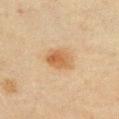follow-up = imaged on a skin check; not biopsied
TBP lesion metrics = a mean CIELAB color near L≈49 a*≈17 b*≈33 and a lesion–skin lightness drop of about 9; a border-irregularity rating of about 2/10 and a color-variation rating of about 4.5/10; a nevus-likeness score of about 100/100 and lesion-presence confidence of about 100/100
site = the chest
subject = male, aged around 45
image = ~15 mm crop, total-body skin-cancer survey
illumination = cross-polarized illumination